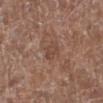Impression: This lesion was catalogued during total-body skin photography and was not selected for biopsy. Background: An algorithmic analysis of the crop reported a border-irregularity index near 2.5/10, a color-variation rating of about 2/10, and peripheral color asymmetry of about 1. And it measured a classifier nevus-likeness of about 0/100 and a lesion-detection confidence of about 100/100. This image is a 15 mm lesion crop taken from a total-body photograph. From the left lower leg. A female subject aged 78 to 82.